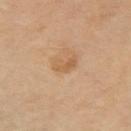Imaged during a routine full-body skin examination; the lesion was not biopsied and no histopathology is available. A close-up tile cropped from a whole-body skin photograph, about 15 mm across. The patient is a male approximately 70 years of age. On the right upper arm. This is a cross-polarized tile. The lesion's longest dimension is about 2.5 mm.